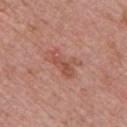Q: Was a biopsy performed?
A: imaged on a skin check; not biopsied
Q: Lesion size?
A: ≈4.5 mm
Q: What kind of image is this?
A: total-body-photography crop, ~15 mm field of view
Q: Who is the patient?
A: male, aged 48 to 52
Q: What is the anatomic site?
A: the front of the torso